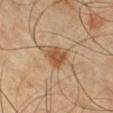Context: From the chest. A male subject, in their 40s. A lesion tile, about 15 mm wide, cut from a 3D total-body photograph.